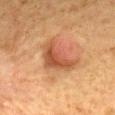The lesion was tiled from a total-body skin photograph and was not biopsied. About 5.5 mm across. Automated image analysis of the tile measured an automated nevus-likeness rating near 95 out of 100 and lesion-presence confidence of about 100/100. Captured under cross-polarized illumination. Located on the mid back. A 15 mm close-up extracted from a 3D total-body photography capture. A male patient, aged 58–62.